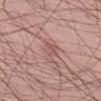Q: Was a biopsy performed?
A: total-body-photography surveillance lesion; no biopsy
Q: Where on the body is the lesion?
A: the left thigh
Q: How large is the lesion?
A: ≈4 mm
Q: Patient demographics?
A: male, in their mid- to late 50s
Q: How was the tile lit?
A: white-light
Q: What kind of image is this?
A: ~15 mm crop, total-body skin-cancer survey